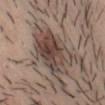follow-up — total-body-photography surveillance lesion; no biopsy | lesion diameter — ≈7 mm | tile lighting — white-light | subject — male, in their mid-20s | anatomic site — the head or neck | imaging modality — total-body-photography crop, ~15 mm field of view.